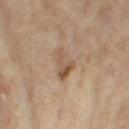<record>
<biopsy_status>not biopsied; imaged during a skin examination</biopsy_status>
<image>
  <source>total-body photography crop</source>
  <field_of_view_mm>15</field_of_view_mm>
</image>
<site>left thigh</site>
<patient>
  <sex>female</sex>
  <age_approx>75</age_approx>
</patient>
</record>